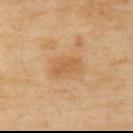{
  "biopsy_status": "not biopsied; imaged during a skin examination",
  "patient": {
    "sex": "female",
    "age_approx": 60
  },
  "automated_metrics": {
    "eccentricity": 0.9,
    "shape_asymmetry": 0.3,
    "vs_skin_darker_L": 6.0,
    "vs_skin_contrast_norm": 5.0,
    "nevus_likeness_0_100": 0,
    "lesion_detection_confidence_0_100": 100
  },
  "site": "upper back",
  "lesion_size": {
    "long_diameter_mm_approx": 3.5
  },
  "lighting": "cross-polarized",
  "image": {
    "source": "total-body photography crop",
    "field_of_view_mm": 15
  }
}